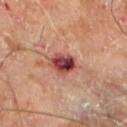* notes — no biopsy performed (imaged during a skin exam)
* patient — male, approximately 70 years of age
* automated metrics — an outline eccentricity of about 0.7 (0 = round, 1 = elongated) and two-axis asymmetry of about 0.2; a border-irregularity rating of about 2/10, a within-lesion color-variation index near 10/10, and a peripheral color-asymmetry measure near 4; an automated nevus-likeness rating near 0 out of 100 and lesion-presence confidence of about 100/100
* imaging modality — total-body-photography crop, ~15 mm field of view
* size — ~3.5 mm (longest diameter)
* location — the left lower leg